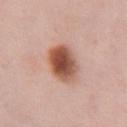Clinical impression:
Recorded during total-body skin imaging; not selected for excision or biopsy.
Acquisition and patient details:
Cropped from a total-body skin-imaging series; the visible field is about 15 mm. The lesion's longest dimension is about 4.5 mm. Captured under white-light illumination. A female patient aged 48 to 52. The lesion is located on the chest.